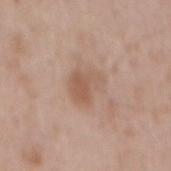biopsy_status: not biopsied; imaged during a skin examination
site: mid back
image:
  source: total-body photography crop
  field_of_view_mm: 15
lighting: white-light
patient:
  sex: male
  age_approx: 50
lesion_size:
  long_diameter_mm_approx: 3.5